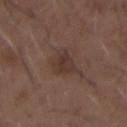Q: What lighting was used for the tile?
A: white-light
Q: What kind of image is this?
A: total-body-photography crop, ~15 mm field of view
Q: Automated lesion metrics?
A: a lesion area of about 6.5 mm² and an eccentricity of roughly 0.7; an average lesion color of about L≈34 a*≈16 b*≈21 (CIELAB) and a lesion-to-skin contrast of about 7 (normalized; higher = more distinct); a nevus-likeness score of about 25/100 and lesion-presence confidence of about 100/100
Q: Where on the body is the lesion?
A: the chest
Q: What are the patient's age and sex?
A: male, roughly 50 years of age
Q: How large is the lesion?
A: ~3.5 mm (longest diameter)